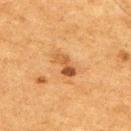follow-up = catalogued during a skin exam; not biopsied
automated lesion analysis = a normalized border contrast of about 7.5; a border-irregularity index near 4/10, a within-lesion color-variation index near 8/10, and radial color variation of about 3
anatomic site = the upper back
patient = male, aged 73 to 77
diameter = about 3.5 mm
imaging modality = ~15 mm crop, total-body skin-cancer survey
illumination = cross-polarized